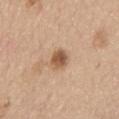{
  "biopsy_status": "not biopsied; imaged during a skin examination"
}